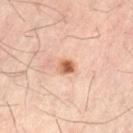Notes:
– notes: imaged on a skin check; not biopsied
– image: ~15 mm crop, total-body skin-cancer survey
– patient: male, in their mid- to late 60s
– location: the left thigh